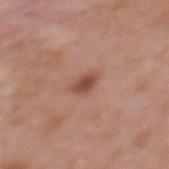The lesion was tiled from a total-body skin photograph and was not biopsied.
A female subject, aged around 35.
Measured at roughly 2.5 mm in maximum diameter.
A 15 mm crop from a total-body photograph taken for skin-cancer surveillance.
Captured under white-light illumination.
The lesion-visualizer software estimated an outline eccentricity of about 0.75 (0 = round, 1 = elongated) and a shape-asymmetry score of about 0.25 (0 = symmetric).
The lesion is on the upper back.